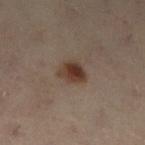Impression:
No biopsy was performed on this lesion — it was imaged during a full skin examination and was not determined to be concerning.
Image and clinical context:
This image is a 15 mm lesion crop taken from a total-body photograph. On the left lower leg. A female subject, in their mid-50s.